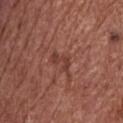Clinical impression: No biopsy was performed on this lesion — it was imaged during a full skin examination and was not determined to be concerning.